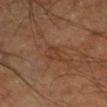Clinical impression:
The lesion was photographed on a routine skin check and not biopsied; there is no pathology result.
Image and clinical context:
Located on the leg. A 15 mm close-up extracted from a 3D total-body photography capture. Approximately 3 mm at its widest. The subject is a male about 65 years old. Imaged with cross-polarized lighting.